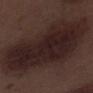| feature | finding |
|---|---|
| notes | total-body-photography surveillance lesion; no biopsy |
| acquisition | 15 mm crop, total-body photography |
| illumination | white-light |
| body site | the left lower leg |
| lesion diameter | ~14.5 mm (longest diameter) |
| subject | male, roughly 70 years of age |